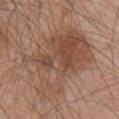  biopsy_status: not biopsied; imaged during a skin examination
  lighting: white-light
  site: left upper arm
  lesion_size:
    long_diameter_mm_approx: 11.0
  image:
    source: total-body photography crop
    field_of_view_mm: 15
  patient:
    sex: male
    age_approx: 45